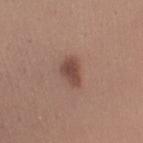Imaged during a routine full-body skin examination; the lesion was not biopsied and no histopathology is available.
On the arm.
The patient is a female in their mid- to late 20s.
A close-up tile cropped from a whole-body skin photograph, about 15 mm across.
The lesion-visualizer software estimated a lesion area of about 5.5 mm², an eccentricity of roughly 0.8, and a symmetry-axis asymmetry near 0.3. The analysis additionally found a mean CIELAB color near L≈46 a*≈20 b*≈24 and a lesion-to-skin contrast of about 8 (normalized; higher = more distinct). The software also gave border irregularity of about 3 on a 0–10 scale and internal color variation of about 2 on a 0–10 scale. It also reported an automated nevus-likeness rating near 95 out of 100 and a lesion-detection confidence of about 100/100.
About 3.5 mm across.
Captured under white-light illumination.